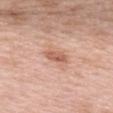Case summary:
* follow-up — no biopsy performed (imaged during a skin exam)
* image — ~15 mm crop, total-body skin-cancer survey
* body site — the upper back
* patient — female, about 65 years old